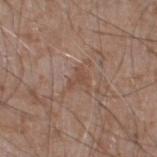The lesion was tiled from a total-body skin photograph and was not biopsied. The subject is a male aged 58 to 62. Imaged with white-light lighting. The lesion is on the left lower leg. A lesion tile, about 15 mm wide, cut from a 3D total-body photograph. An algorithmic analysis of the crop reported a lesion area of about 3 mm², an outline eccentricity of about 0.65 (0 = round, 1 = elongated), and a shape-asymmetry score of about 0.55 (0 = symmetric). The software also gave a mean CIELAB color near L≈48 a*≈18 b*≈27 and roughly 6 lightness units darker than nearby skin. The software also gave a within-lesion color-variation index near 0.5/10. It also reported a lesion-detection confidence of about 70/100. The recorded lesion diameter is about 2.5 mm.